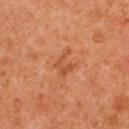Captured during whole-body skin photography for melanoma surveillance; the lesion was not biopsied. The lesion is located on the back. The patient is a female aged approximately 40. Automated tile analysis of the lesion measured an eccentricity of roughly 0.6 and a symmetry-axis asymmetry near 0.45. The analysis additionally found a nevus-likeness score of about 0/100 and a lesion-detection confidence of about 100/100. A 15 mm close-up extracted from a 3D total-body photography capture. Longest diameter approximately 3 mm.